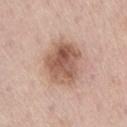Q: Was this lesion biopsied?
A: catalogued during a skin exam; not biopsied
Q: What kind of image is this?
A: 15 mm crop, total-body photography
Q: What is the anatomic site?
A: the right thigh
Q: Patient demographics?
A: male, aged around 75
Q: What lighting was used for the tile?
A: white-light
Q: What is the lesion's diameter?
A: ≈5.5 mm